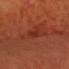Captured during whole-body skin photography for melanoma surveillance; the lesion was not biopsied.
On the head or neck.
A male patient roughly 60 years of age.
A 15 mm close-up extracted from a 3D total-body photography capture.
Automated tile analysis of the lesion measured a lesion color around L≈31 a*≈31 b*≈32 in CIELAB. The analysis additionally found a border-irregularity rating of about 3/10, a color-variation rating of about 0/10, and peripheral color asymmetry of about 0.
Measured at roughly 3 mm in maximum diameter.